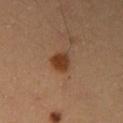{
  "biopsy_status": "not biopsied; imaged during a skin examination",
  "site": "right upper arm",
  "lesion_size": {
    "long_diameter_mm_approx": 2.5
  },
  "automated_metrics": {
    "area_mm2_approx": 5.5,
    "shape_asymmetry": 0.25,
    "cielab_L": 39,
    "cielab_a": 21,
    "cielab_b": 33,
    "vs_skin_darker_L": 11.0,
    "vs_skin_contrast_norm": 10.0,
    "border_irregularity_0_10": 2.0,
    "color_variation_0_10": 3.5
  },
  "lighting": "cross-polarized",
  "patient": {
    "sex": "female",
    "age_approx": 35
  },
  "image": {
    "source": "total-body photography crop",
    "field_of_view_mm": 15
  }
}